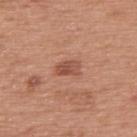Part of a total-body skin-imaging series; this lesion was reviewed on a skin check and was not flagged for biopsy. A male patient aged 73–77. This image is a 15 mm lesion crop taken from a total-body photograph. The recorded lesion diameter is about 2.5 mm. Located on the upper back.